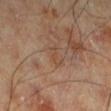| key | value |
|---|---|
| image source | 15 mm crop, total-body photography |
| location | the left lower leg |
| diameter | ≈3 mm |
| TBP lesion metrics | a shape eccentricity near 0.9 and a symmetry-axis asymmetry near 0.4; roughly 5 lightness units darker than nearby skin and a lesion-to-skin contrast of about 4.5 (normalized; higher = more distinct); a peripheral color-asymmetry measure near 0; lesion-presence confidence of about 100/100 |
| patient | male, approximately 70 years of age |
| tile lighting | cross-polarized illumination |